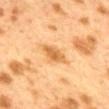{"biopsy_status": "not biopsied; imaged during a skin examination", "lesion_size": {"long_diameter_mm_approx": 3.0}, "automated_metrics": {"vs_skin_darker_L": 10.0, "vs_skin_contrast_norm": 7.5}, "site": "mid back", "lighting": "cross-polarized", "image": {"source": "total-body photography crop", "field_of_view_mm": 15}, "patient": {"sex": "female", "age_approx": 40}}